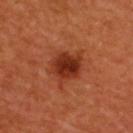image:
  source: total-body photography crop
  field_of_view_mm: 15
lighting: cross-polarized
patient:
  sex: female
  age_approx: 45
site: upper back
lesion_size:
  long_diameter_mm_approx: 4.0
automated_metrics:
  cielab_L: 34
  cielab_a: 31
  cielab_b: 34
  border_irregularity_0_10: 2.0
  color_variation_0_10: 4.0
  peripheral_color_asymmetry: 1.5
  nevus_likeness_0_100: 95
  lesion_detection_confidence_0_100: 100